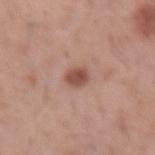This lesion was catalogued during total-body skin photography and was not selected for biopsy. The tile uses white-light illumination. A roughly 15 mm field-of-view crop from a total-body skin photograph. A male subject, aged approximately 40. About 2.5 mm across. The lesion is located on the arm.